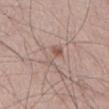Background: Located on the abdomen. A lesion tile, about 15 mm wide, cut from a 3D total-body photograph. Longest diameter approximately 4 mm. A male subject, aged around 60. The tile uses white-light illumination.